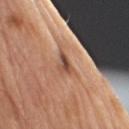<case>
  <biopsy_status>not biopsied; imaged during a skin examination</biopsy_status>
  <lighting>white-light</lighting>
  <patient>
    <sex>male</sex>
    <age_approx>70</age_approx>
  </patient>
  <lesion_size>
    <long_diameter_mm_approx>2.5</long_diameter_mm_approx>
  </lesion_size>
  <automated_metrics>
    <cielab_L>50</cielab_L>
    <cielab_a>22</cielab_a>
    <cielab_b>30</cielab_b>
    <vs_skin_darker_L>12.0</vs_skin_darker_L>
    <vs_skin_contrast_norm>8.5</vs_skin_contrast_norm>
    <border_irregularity_0_10>4.0</border_irregularity_0_10>
    <peripheral_color_asymmetry>2.0</peripheral_color_asymmetry>
    <nevus_likeness_0_100>0</nevus_likeness_0_100>
    <lesion_detection_confidence_0_100>100</lesion_detection_confidence_0_100>
  </automated_metrics>
  <site>left upper arm</site>
  <image>
    <source>total-body photography crop</source>
    <field_of_view_mm>15</field_of_view_mm>
  </image>
</case>